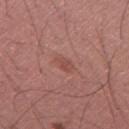follow-up — total-body-photography surveillance lesion; no biopsy | subject — male, approximately 45 years of age | image — ~15 mm crop, total-body skin-cancer survey | location — the right thigh | automated metrics — an area of roughly 3 mm², an outline eccentricity of about 0.85 (0 = round, 1 = elongated), and a symmetry-axis asymmetry near 0.35; a border-irregularity rating of about 3.5/10, a within-lesion color-variation index near 0.5/10, and a peripheral color-asymmetry measure near 0.